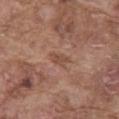Findings:
– follow-up: total-body-photography surveillance lesion; no biopsy
– body site: the abdomen
– lesion diameter: about 2.5 mm
– subject: male, aged approximately 75
– illumination: white-light illumination
– imaging modality: 15 mm crop, total-body photography